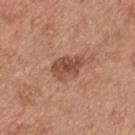Q: Was a biopsy performed?
A: catalogued during a skin exam; not biopsied
Q: How large is the lesion?
A: ~4 mm (longest diameter)
Q: What kind of image is this?
A: ~15 mm crop, total-body skin-cancer survey
Q: Automated lesion metrics?
A: a lesion area of about 7 mm², an outline eccentricity of about 0.8 (0 = round, 1 = elongated), and two-axis asymmetry of about 0.25; a nevus-likeness score of about 25/100
Q: Where on the body is the lesion?
A: the chest
Q: Illumination type?
A: white-light illumination
Q: What are the patient's age and sex?
A: male, roughly 55 years of age